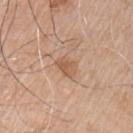notes — total-body-photography surveillance lesion; no biopsy | patient — male, aged 73–77 | lighting — white-light | acquisition — ~15 mm tile from a whole-body skin photo | lesion diameter — ~2.5 mm (longest diameter) | body site — the chest.